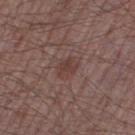notes: catalogued during a skin exam; not biopsied
automated metrics: a border-irregularity index near 2.5/10, a within-lesion color-variation index near 2/10, and a peripheral color-asymmetry measure near 0.5
location: the leg
imaging modality: 15 mm crop, total-body photography
lesion diameter: ≈3 mm
patient: male, aged 63–67